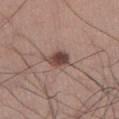notes: no biopsy performed (imaged during a skin exam) | image source: total-body-photography crop, ~15 mm field of view | patient: male, in their mid-30s | illumination: white-light illumination | body site: the right lower leg.